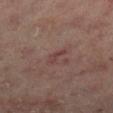No biopsy was performed on this lesion — it was imaged during a full skin examination and was not determined to be concerning. A male patient, approximately 65 years of age. A region of skin cropped from a whole-body photographic capture, roughly 15 mm wide. Captured under cross-polarized illumination. Measured at roughly 2.5 mm in maximum diameter. The lesion is on the left lower leg.